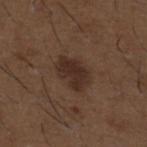• location — the upper back
• subject — male, about 50 years old
• image — ~15 mm crop, total-body skin-cancer survey
• tile lighting — white-light illumination